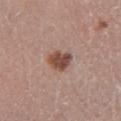Impression: The lesion was tiled from a total-body skin photograph and was not biopsied. Clinical summary: Captured under white-light illumination. The lesion is located on the left lower leg. The recorded lesion diameter is about 3 mm. A close-up tile cropped from a whole-body skin photograph, about 15 mm across. The lesion-visualizer software estimated a lesion area of about 6.5 mm², an outline eccentricity of about 0.65 (0 = round, 1 = elongated), and two-axis asymmetry of about 0.25. And it measured a classifier nevus-likeness of about 95/100. A male patient, aged 73–77.